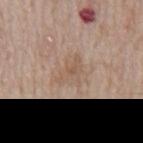Clinical impression:
The lesion was tiled from a total-body skin photograph and was not biopsied.
Background:
Cropped from a total-body skin-imaging series; the visible field is about 15 mm. A male patient aged 63–67. Located on the back.